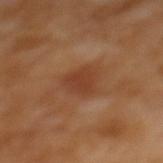Clinical impression:
The lesion was tiled from a total-body skin photograph and was not biopsied.
Context:
The recorded lesion diameter is about 3.5 mm. A 15 mm close-up tile from a total-body photography series done for melanoma screening. On the upper back. A female subject, aged around 55. This is a cross-polarized tile.